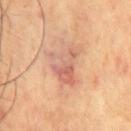The lesion was tiled from a total-body skin photograph and was not biopsied.
This image is a 15 mm lesion crop taken from a total-body photograph.
Measured at roughly 4.5 mm in maximum diameter.
The patient is a male in their mid- to late 60s.
From the front of the torso.
The lesion-visualizer software estimated a footprint of about 11 mm² and an outline eccentricity of about 0.75 (0 = round, 1 = elongated). The analysis additionally found a nevus-likeness score of about 0/100 and lesion-presence confidence of about 100/100.